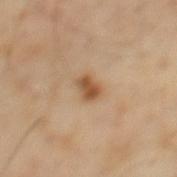The lesion is on the mid back. A 15 mm close-up tile from a total-body photography series done for melanoma screening. A male patient, aged 38–42.